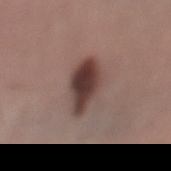This lesion was catalogued during total-body skin photography and was not selected for biopsy.
The subject is a female aged around 55.
Captured under white-light illumination.
A lesion tile, about 15 mm wide, cut from a 3D total-body photograph.
Approximately 5 mm at its widest.
The lesion is on the left lower leg.
An algorithmic analysis of the crop reported a lesion color around L≈39 a*≈18 b*≈20 in CIELAB, about 15 CIELAB-L* units darker than the surrounding skin, and a lesion-to-skin contrast of about 11.5 (normalized; higher = more distinct). The software also gave a border-irregularity index near 2/10 and a color-variation rating of about 6/10. The software also gave a classifier nevus-likeness of about 95/100 and lesion-presence confidence of about 100/100.